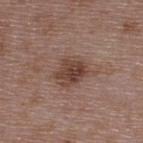{"lighting": "white-light", "patient": {"sex": "male", "age_approx": 50}, "site": "upper back", "lesion_size": {"long_diameter_mm_approx": 4.0}, "image": {"source": "total-body photography crop", "field_of_view_mm": 15}}